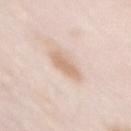| key | value |
|---|---|
| follow-up | total-body-photography surveillance lesion; no biopsy |
| lesion size | ≈3.5 mm |
| patient | female, aged 63–67 |
| image-analysis metrics | a within-lesion color-variation index near 1.5/10 and peripheral color asymmetry of about 0.5 |
| site | the chest |
| image source | 15 mm crop, total-body photography |
| lighting | white-light illumination |